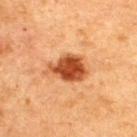Part of a total-body skin-imaging series; this lesion was reviewed on a skin check and was not flagged for biopsy.
Automated tile analysis of the lesion measured a mean CIELAB color near L≈42 a*≈26 b*≈35, about 14 CIELAB-L* units darker than the surrounding skin, and a lesion-to-skin contrast of about 11 (normalized; higher = more distinct).
A lesion tile, about 15 mm wide, cut from a 3D total-body photograph.
A male subject, aged around 50.
This is a cross-polarized tile.
On the upper back.